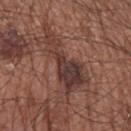The lesion was photographed on a routine skin check and not biopsied; there is no pathology result.
On the left upper arm.
Cropped from a total-body skin-imaging series; the visible field is about 15 mm.
The subject is a male in their mid-60s.